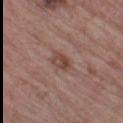{
  "biopsy_status": "not biopsied; imaged during a skin examination",
  "lesion_size": {
    "long_diameter_mm_approx": 3.5
  },
  "image": {
    "source": "total-body photography crop",
    "field_of_view_mm": 15
  },
  "lighting": "white-light",
  "site": "left thigh",
  "patient": {
    "sex": "male",
    "age_approx": 65
  },
  "automated_metrics": {
    "cielab_L": 45,
    "cielab_a": 20,
    "cielab_b": 24,
    "vs_skin_darker_L": 9.0,
    "vs_skin_contrast_norm": 7.0,
    "border_irregularity_0_10": 3.0,
    "color_variation_0_10": 4.5,
    "peripheral_color_asymmetry": 1.5
  }
}